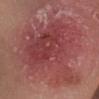No biopsy was performed on this lesion — it was imaged during a full skin examination and was not determined to be concerning. Measured at roughly 13 mm in maximum diameter. This is a white-light tile. Cropped from a total-body skin-imaging series; the visible field is about 15 mm. The patient is a female aged 28 to 32. Located on the head or neck. The total-body-photography lesion software estimated an automated nevus-likeness rating near 0 out of 100 and lesion-presence confidence of about 100/100.